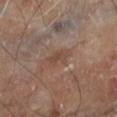biopsy_status: not biopsied; imaged during a skin examination
automated_metrics:
  shape_asymmetry: 0.4
  cielab_L: 43
  cielab_a: 17
  cielab_b: 25
  vs_skin_contrast_norm: 5.5
image:
  source: total-body photography crop
  field_of_view_mm: 15
patient:
  sex: male
  age_approx: 70
site: left lower leg
lighting: cross-polarized
lesion_size:
  long_diameter_mm_approx: 2.5